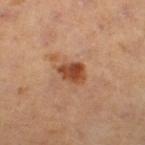acquisition: 15 mm crop, total-body photography
site: the right thigh
subject: female, roughly 40 years of age
lighting: cross-polarized
image-analysis metrics: a mean CIELAB color near L≈46 a*≈26 b*≈35, a lesion–skin lightness drop of about 13, and a lesion-to-skin contrast of about 10 (normalized; higher = more distinct); a nevus-likeness score of about 95/100
diameter: ≈3 mm